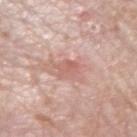  site: left forearm
  lighting: white-light
  lesion_size:
    long_diameter_mm_approx: 2.5
  automated_metrics:
    area_mm2_approx: 4.0
    eccentricity: 0.45
    shape_asymmetry: 0.25
    vs_skin_darker_L: 8.0
    vs_skin_contrast_norm: 5.0
    border_irregularity_0_10: 3.0
    color_variation_0_10: 2.5
    peripheral_color_asymmetry: 1.0
    lesion_detection_confidence_0_100: 100
  patient:
    sex: male
    age_approx: 75
  image:
    source: total-body photography crop
    field_of_view_mm: 15